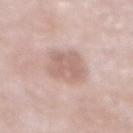Part of a total-body skin-imaging series; this lesion was reviewed on a skin check and was not flagged for biopsy.
An algorithmic analysis of the crop reported an average lesion color of about L≈63 a*≈18 b*≈24 (CIELAB) and roughly 9 lightness units darker than nearby skin. The software also gave border irregularity of about 2 on a 0–10 scale, a color-variation rating of about 2.5/10, and a peripheral color-asymmetry measure near 1.
This is a white-light tile.
This image is a 15 mm lesion crop taken from a total-body photograph.
A male subject, in their mid-50s.
Longest diameter approximately 4.5 mm.
On the abdomen.